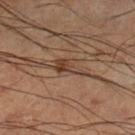No biopsy was performed on this lesion — it was imaged during a full skin examination and was not determined to be concerning. This is a cross-polarized tile. A close-up tile cropped from a whole-body skin photograph, about 15 mm across. The lesion is located on the left lower leg. The lesion's longest dimension is about 3.5 mm. Automated tile analysis of the lesion measured an area of roughly 4 mm² and an outline eccentricity of about 0.9 (0 = round, 1 = elongated). The software also gave a mean CIELAB color near L≈38 a*≈18 b*≈27, roughly 9 lightness units darker than nearby skin, and a lesion-to-skin contrast of about 8 (normalized; higher = more distinct). It also reported a classifier nevus-likeness of about 25/100 and a lesion-detection confidence of about 95/100. The subject is a male about 60 years old.